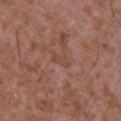A male subject, aged 43 to 47. The lesion is on the chest. The tile uses white-light illumination. A roughly 15 mm field-of-view crop from a total-body skin photograph. Longest diameter approximately 2.5 mm.